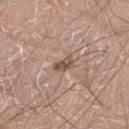A region of skin cropped from a whole-body photographic capture, roughly 15 mm wide. The lesion is on the leg. A male subject, in their 80s.